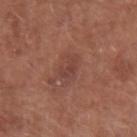Impression: The lesion was photographed on a routine skin check and not biopsied; there is no pathology result. Clinical summary: The subject is a male in their 60s. Automated tile analysis of the lesion measured a border-irregularity rating of about 4/10 and a peripheral color-asymmetry measure near 0. The lesion is located on the right upper arm. A close-up tile cropped from a whole-body skin photograph, about 15 mm across.